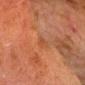Impression:
This lesion was catalogued during total-body skin photography and was not selected for biopsy.
Context:
The tile uses cross-polarized illumination. A male subject, roughly 80 years of age. The lesion is located on the head or neck. A roughly 15 mm field-of-view crop from a total-body skin photograph.